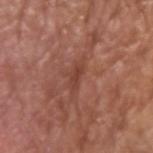| field | value |
|---|---|
| subject | male, aged around 75 |
| lighting | white-light |
| imaging modality | ~15 mm tile from a whole-body skin photo |
| diameter | about 2.5 mm |
| site | the left upper arm |
| automated lesion analysis | border irregularity of about 4 on a 0–10 scale, internal color variation of about 0 on a 0–10 scale, and peripheral color asymmetry of about 0 |